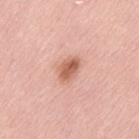workup = catalogued during a skin exam; not biopsied | anatomic site = the lower back | lesion diameter = about 3 mm | tile lighting = white-light illumination | automated lesion analysis = a lesion area of about 5 mm², a shape eccentricity near 0.75, and two-axis asymmetry of about 0.25; a classifier nevus-likeness of about 95/100 and lesion-presence confidence of about 100/100 | acquisition = ~15 mm tile from a whole-body skin photo | subject = female, in their mid- to late 60s.